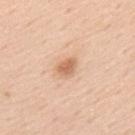No biopsy was performed on this lesion — it was imaged during a full skin examination and was not determined to be concerning.